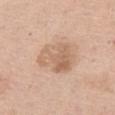Imaged during a routine full-body skin examination; the lesion was not biopsied and no histopathology is available. Cropped from a whole-body photographic skin survey; the tile spans about 15 mm. The patient is a female aged 63–67. The lesion is on the right thigh.